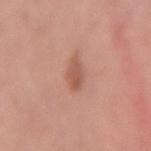Q: Is there a histopathology result?
A: catalogued during a skin exam; not biopsied
Q: What is the lesion's diameter?
A: ~3 mm (longest diameter)
Q: What are the patient's age and sex?
A: female, aged 33–37
Q: What is the imaging modality?
A: ~15 mm tile from a whole-body skin photo
Q: Lesion location?
A: the arm